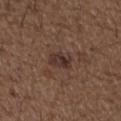Imaged during a routine full-body skin examination; the lesion was not biopsied and no histopathology is available.
A male patient approximately 50 years of age.
Longest diameter approximately 3 mm.
The lesion is on the left upper arm.
A 15 mm crop from a total-body photograph taken for skin-cancer surveillance.
An algorithmic analysis of the crop reported roughly 8 lightness units darker than nearby skin and a normalized border contrast of about 8.
Imaged with white-light lighting.